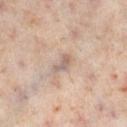Recorded during total-body skin imaging; not selected for excision or biopsy.
Captured under cross-polarized illumination.
From the right lower leg.
A female subject aged 48 to 52.
A region of skin cropped from a whole-body photographic capture, roughly 15 mm wide.
An algorithmic analysis of the crop reported a mean CIELAB color near L≈59 a*≈14 b*≈23, about 9 CIELAB-L* units darker than the surrounding skin, and a normalized lesion–skin contrast near 6. It also reported a nevus-likeness score of about 0/100 and lesion-presence confidence of about 60/100.
The lesion's longest dimension is about 3 mm.